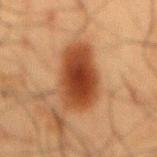Case summary:
• notes · catalogued during a skin exam; not biopsied
• lesion size · about 7 mm
• automated lesion analysis · a color-variation rating of about 4.5/10 and radial color variation of about 1
• imaging modality · total-body-photography crop, ~15 mm field of view
• body site · the mid back
• patient · male, aged approximately 60